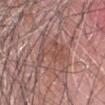Captured during whole-body skin photography for melanoma surveillance; the lesion was not biopsied.
Located on the head or neck.
Automated tile analysis of the lesion measured an area of roughly 9.5 mm², a shape eccentricity near 0.95, and a symmetry-axis asymmetry near 0.55. The analysis additionally found a lesion color around L≈50 a*≈22 b*≈24 in CIELAB, about 7 CIELAB-L* units darker than the surrounding skin, and a normalized border contrast of about 5.5. The analysis additionally found internal color variation of about 3 on a 0–10 scale and peripheral color asymmetry of about 1. The analysis additionally found a classifier nevus-likeness of about 0/100 and a detector confidence of about 75 out of 100 that the crop contains a lesion.
The lesion's longest dimension is about 6 mm.
The tile uses white-light illumination.
A male subject, aged 78–82.
A 15 mm close-up extracted from a 3D total-body photography capture.